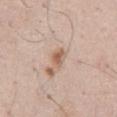Impression: Part of a total-body skin-imaging series; this lesion was reviewed on a skin check and was not flagged for biopsy. Acquisition and patient details: About 3 mm across. A 15 mm crop from a total-body photograph taken for skin-cancer surveillance. The total-body-photography lesion software estimated a lesion color around L≈60 a*≈19 b*≈29 in CIELAB, a lesion–skin lightness drop of about 10, and a lesion-to-skin contrast of about 7 (normalized; higher = more distinct). It also reported border irregularity of about 2.5 on a 0–10 scale, a color-variation rating of about 2.5/10, and radial color variation of about 1. A male patient, aged approximately 55. Imaged with white-light lighting. On the abdomen.